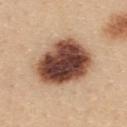Assessment:
This lesion was catalogued during total-body skin photography and was not selected for biopsy.
Image and clinical context:
The lesion is on the back. About 7.5 mm across. A female patient, aged around 25. The tile uses white-light illumination. Cropped from a whole-body photographic skin survey; the tile spans about 15 mm.